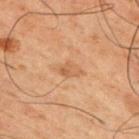follow-up: no biopsy performed (imaged during a skin exam)
acquisition: ~15 mm crop, total-body skin-cancer survey
TBP lesion metrics: a classifier nevus-likeness of about 0/100 and a lesion-detection confidence of about 100/100
subject: male, about 50 years old
lesion diameter: ~3.5 mm (longest diameter)
location: the chest
illumination: cross-polarized illumination